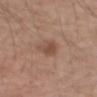| feature | finding |
|---|---|
| workup | imaged on a skin check; not biopsied |
| image | ~15 mm crop, total-body skin-cancer survey |
| body site | the left forearm |
| automated lesion analysis | a footprint of about 6.5 mm², an eccentricity of roughly 0.5, and two-axis asymmetry of about 0.25; a mean CIELAB color near L≈49 a*≈19 b*≈28 and a normalized border contrast of about 6 |
| lesion size | ≈3 mm |
| tile lighting | white-light illumination |
| subject | male, aged around 65 |